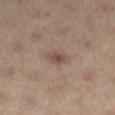workup: imaged on a skin check; not biopsied
subject: female, aged 38–42
illumination: cross-polarized
diameter: ≈2.5 mm
TBP lesion metrics: a mean CIELAB color near L≈48 a*≈18 b*≈21, roughly 9 lightness units darker than nearby skin, and a normalized lesion–skin contrast near 7; a border-irregularity index near 2/10, a within-lesion color-variation index near 2.5/10, and peripheral color asymmetry of about 1; a nevus-likeness score of about 10/100 and lesion-presence confidence of about 100/100
site: the right leg
acquisition: 15 mm crop, total-body photography